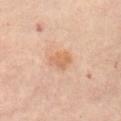Impression: The lesion was photographed on a routine skin check and not biopsied; there is no pathology result. Clinical summary: On the abdomen. A roughly 15 mm field-of-view crop from a total-body skin photograph. Captured under cross-polarized illumination. The lesion-visualizer software estimated a lesion area of about 4.5 mm², an eccentricity of roughly 0.65, and a shape-asymmetry score of about 0.3 (0 = symmetric). It also reported a nevus-likeness score of about 20/100 and a lesion-detection confidence of about 100/100. A female subject, in their mid- to late 60s. Measured at roughly 3 mm in maximum diameter.